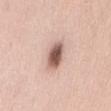Clinical impression: No biopsy was performed on this lesion — it was imaged during a full skin examination and was not determined to be concerning. Context: The subject is a female aged around 60. The lesion is on the lower back. This is a white-light tile. Cropped from a whole-body photographic skin survey; the tile spans about 15 mm.